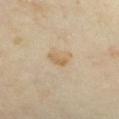Background:
Captured under cross-polarized illumination. On the front of the torso. A 15 mm crop from a total-body photograph taken for skin-cancer surveillance. A female subject, about 40 years old.